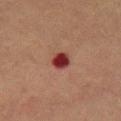Part of a total-body skin-imaging series; this lesion was reviewed on a skin check and was not flagged for biopsy.
Cropped from a whole-body photographic skin survey; the tile spans about 15 mm.
Captured under cross-polarized illumination.
From the chest.
A female subject approximately 60 years of age.
Approximately 2.5 mm at its widest.